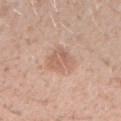biopsy status = no biopsy performed (imaged during a skin exam)
image source = 15 mm crop, total-body photography
anatomic site = the right forearm
patient = male, aged approximately 30